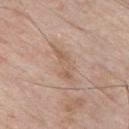Q: Was this lesion biopsied?
A: total-body-photography surveillance lesion; no biopsy
Q: Where on the body is the lesion?
A: the chest
Q: Patient demographics?
A: male, approximately 55 years of age
Q: Illumination type?
A: white-light illumination
Q: What is the imaging modality?
A: ~15 mm tile from a whole-body skin photo
Q: Automated lesion metrics?
A: a lesion area of about 5.5 mm², a shape eccentricity near 0.95, and a symmetry-axis asymmetry near 0.5